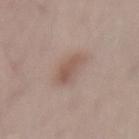Assessment:
The lesion was photographed on a routine skin check and not biopsied; there is no pathology result.
Background:
From the back. The tile uses white-light illumination. The subject is a male aged around 55. Cropped from a total-body skin-imaging series; the visible field is about 15 mm. About 4 mm across.